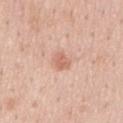This lesion was catalogued during total-body skin photography and was not selected for biopsy.
Located on the chest.
Approximately 2.5 mm at its widest.
Imaged with white-light lighting.
A male subject about 50 years old.
This image is a 15 mm lesion crop taken from a total-body photograph.